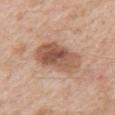The lesion was tiled from a total-body skin photograph and was not biopsied.
A male patient aged approximately 60.
The tile uses white-light illumination.
This image is a 15 mm lesion crop taken from a total-body photograph.
The lesion is on the left upper arm.
Approximately 6.5 mm at its widest.